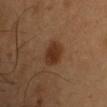Acquisition and patient details: The lesion is on the chest. The subject is a male aged 53 to 57. The total-body-photography lesion software estimated a color-variation rating of about 3/10. The analysis additionally found a classifier nevus-likeness of about 95/100 and lesion-presence confidence of about 100/100. This is a cross-polarized tile. Cropped from a total-body skin-imaging series; the visible field is about 15 mm. The lesion's longest dimension is about 3.5 mm.